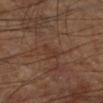  biopsy_status: not biopsied; imaged during a skin examination
  site: leg
  lighting: cross-polarized
  patient:
    sex: male
    age_approx: 60
  image:
    source: total-body photography crop
    field_of_view_mm: 15